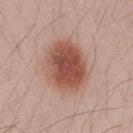This lesion was catalogued during total-body skin photography and was not selected for biopsy.
Imaged with white-light lighting.
This image is a 15 mm lesion crop taken from a total-body photograph.
The recorded lesion diameter is about 6 mm.
Automated tile analysis of the lesion measured an outline eccentricity of about 0.6 (0 = round, 1 = elongated) and two-axis asymmetry of about 0.1. The software also gave a border-irregularity index near 1/10, a color-variation rating of about 6/10, and radial color variation of about 1.5.
The lesion is on the right thigh.
The patient is a male approximately 30 years of age.